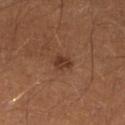- follow-up — no biopsy performed (imaged during a skin exam)
- site — the left lower leg
- patient — male, approximately 40 years of age
- image source — 15 mm crop, total-body photography
- lighting — cross-polarized illumination
- diameter — ≈2.5 mm
- image-analysis metrics — a border-irregularity rating of about 3/10, internal color variation of about 2.5 on a 0–10 scale, and peripheral color asymmetry of about 1